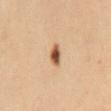follow-up = catalogued during a skin exam; not biopsied
acquisition = ~15 mm tile from a whole-body skin photo
body site = the mid back
lesion size = about 2.5 mm
patient = female, in their 30s